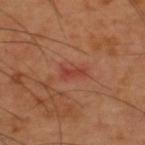Recorded during total-body skin imaging; not selected for excision or biopsy. Located on the upper back. A male subject, aged 58 to 62. A roughly 15 mm field-of-view crop from a total-body skin photograph. The recorded lesion diameter is about 3 mm.